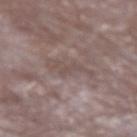body site = the left forearm
TBP lesion metrics = a mean CIELAB color near L≈49 a*≈14 b*≈18, about 6 CIELAB-L* units darker than the surrounding skin, and a normalized lesion–skin contrast near 4.5; border irregularity of about 6.5 on a 0–10 scale, a color-variation rating of about 1.5/10, and peripheral color asymmetry of about 0.5; a nevus-likeness score of about 0/100 and a detector confidence of about 55 out of 100 that the crop contains a lesion
tile lighting = white-light illumination
acquisition = total-body-photography crop, ~15 mm field of view
size = ≈4 mm
patient = male, aged 63–67